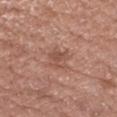| feature | finding |
|---|---|
| workup | imaged on a skin check; not biopsied |
| location | the left forearm |
| acquisition | 15 mm crop, total-body photography |
| automated metrics | an area of roughly 5 mm² and a shape eccentricity near 0.4; a classifier nevus-likeness of about 0/100 and lesion-presence confidence of about 100/100 |
| lighting | white-light |
| subject | male, aged 63–67 |